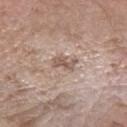{
  "biopsy_status": "not biopsied; imaged during a skin examination"
}